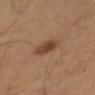{
  "biopsy_status": "not biopsied; imaged during a skin examination",
  "site": "right upper arm",
  "lighting": "cross-polarized",
  "automated_metrics": {
    "border_irregularity_0_10": 2.0,
    "color_variation_0_10": 3.5,
    "peripheral_color_asymmetry": 1.0,
    "lesion_detection_confidence_0_100": 100
  },
  "lesion_size": {
    "long_diameter_mm_approx": 4.0
  },
  "image": {
    "source": "total-body photography crop",
    "field_of_view_mm": 15
  },
  "patient": {
    "sex": "male",
    "age_approx": 50
  }
}